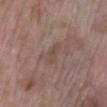notes = total-body-photography surveillance lesion; no biopsy | lesion diameter = about 2.5 mm | patient = female, aged 68–72 | image source = total-body-photography crop, ~15 mm field of view | anatomic site = the right lower leg | illumination = white-light illumination.